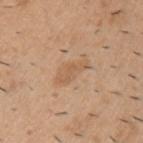follow-up: total-body-photography surveillance lesion; no biopsy | size: about 4.5 mm | tile lighting: white-light | acquisition: total-body-photography crop, ~15 mm field of view | body site: the left upper arm | patient: male, roughly 40 years of age.